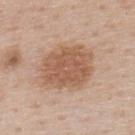The lesion was tiled from a total-body skin photograph and was not biopsied.
The lesion's longest dimension is about 6 mm.
A male patient approximately 60 years of age.
From the upper back.
This is a white-light tile.
A 15 mm close-up tile from a total-body photography series done for melanoma screening.